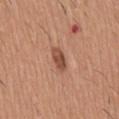The lesion was photographed on a routine skin check and not biopsied; there is no pathology result. The subject is a male in their 60s. Imaged with white-light lighting. This image is a 15 mm lesion crop taken from a total-body photograph. Measured at roughly 3 mm in maximum diameter. On the mid back.